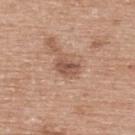Imaged during a routine full-body skin examination; the lesion was not biopsied and no histopathology is available. Automated tile analysis of the lesion measured a footprint of about 4.5 mm², an eccentricity of roughly 0.7, and two-axis asymmetry of about 0.3. And it measured an average lesion color of about L≈53 a*≈21 b*≈28 (CIELAB), about 11 CIELAB-L* units darker than the surrounding skin, and a normalized lesion–skin contrast near 7.5. Located on the upper back. A lesion tile, about 15 mm wide, cut from a 3D total-body photograph. Captured under white-light illumination. A female subject aged 58–62. Approximately 2.5 mm at its widest.